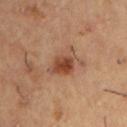The lesion was photographed on a routine skin check and not biopsied; there is no pathology result. Longest diameter approximately 3.5 mm. A male patient, in their mid-50s. An algorithmic analysis of the crop reported a mean CIELAB color near L≈45 a*≈22 b*≈31, a lesion–skin lightness drop of about 11, and a lesion-to-skin contrast of about 8.5 (normalized; higher = more distinct). It also reported a nevus-likeness score of about 90/100 and lesion-presence confidence of about 100/100. Cropped from a total-body skin-imaging series; the visible field is about 15 mm. On the chest.